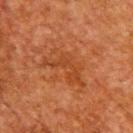{
  "lighting": "cross-polarized",
  "patient": {
    "sex": "male",
    "age_approx": 60
  },
  "site": "upper back",
  "image": {
    "source": "total-body photography crop",
    "field_of_view_mm": 15
  },
  "lesion_size": {
    "long_diameter_mm_approx": 5.5
  }
}